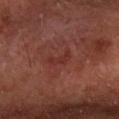notes — total-body-photography surveillance lesion; no biopsy
lesion diameter — ~3 mm (longest diameter)
patient — male, aged 58–62
acquisition — ~15 mm crop, total-body skin-cancer survey
location — the right forearm